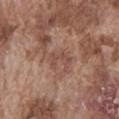Clinical impression: Part of a total-body skin-imaging series; this lesion was reviewed on a skin check and was not flagged for biopsy. Clinical summary: A male subject, in their mid-70s. The total-body-photography lesion software estimated an area of roughly 4.5 mm², a shape eccentricity near 0.75, and a shape-asymmetry score of about 0.4 (0 = symmetric). It also reported a border-irregularity index near 5/10, a color-variation rating of about 1.5/10, and peripheral color asymmetry of about 0.5. Captured under white-light illumination. Cropped from a whole-body photographic skin survey; the tile spans about 15 mm. Located on the abdomen. About 3 mm across.